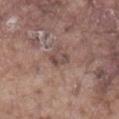{"biopsy_status": "not biopsied; imaged during a skin examination", "automated_metrics": {"area_mm2_approx": 3.5, "border_irregularity_0_10": 2.5, "color_variation_0_10": 3.5, "peripheral_color_asymmetry": 1.0}, "patient": {"sex": "male", "age_approx": 75}, "site": "abdomen", "image": {"source": "total-body photography crop", "field_of_view_mm": 15}}